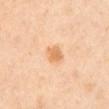Clinical impression:
Part of a total-body skin-imaging series; this lesion was reviewed on a skin check and was not flagged for biopsy.
Clinical summary:
On the abdomen. Imaged with cross-polarized lighting. The subject is a female aged 53 to 57. A lesion tile, about 15 mm wide, cut from a 3D total-body photograph. Longest diameter approximately 2.5 mm.